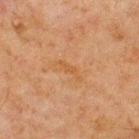Recorded during total-body skin imaging; not selected for excision or biopsy. On the upper back. A male subject, approximately 70 years of age. A roughly 15 mm field-of-view crop from a total-body skin photograph. Automated tile analysis of the lesion measured a color-variation rating of about 0/10 and peripheral color asymmetry of about 0. Captured under cross-polarized illumination. Measured at roughly 3.5 mm in maximum diameter.